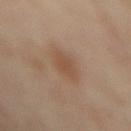workup = no biopsy performed (imaged during a skin exam); subject = female, aged 53–57; lesion diameter = about 3.5 mm; image source = ~15 mm tile from a whole-body skin photo; site = the mid back; lighting = cross-polarized.